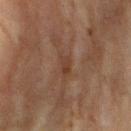The recorded lesion diameter is about 2.5 mm.
This image is a 15 mm lesion crop taken from a total-body photograph.
On the right upper arm.
A female subject, aged approximately 80.
Captured under cross-polarized illumination.